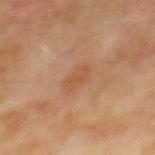Impression: Imaged during a routine full-body skin examination; the lesion was not biopsied and no histopathology is available. Background: A 15 mm close-up tile from a total-body photography series done for melanoma screening. The lesion's longest dimension is about 3 mm. This is a cross-polarized tile. Located on the mid back. A male patient, aged around 75.